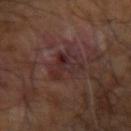Impression:
Captured during whole-body skin photography for melanoma surveillance; the lesion was not biopsied.
Image and clinical context:
A male subject, aged approximately 60. Cropped from a whole-body photographic skin survey; the tile spans about 15 mm. The lesion is on the right arm.